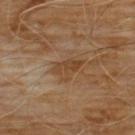Q: Is there a histopathology result?
A: catalogued during a skin exam; not biopsied
Q: How was this image acquired?
A: ~15 mm crop, total-body skin-cancer survey
Q: Where on the body is the lesion?
A: the chest
Q: Lesion size?
A: ~3 mm (longest diameter)
Q: Who is the patient?
A: male, aged 58 to 62
Q: Illumination type?
A: cross-polarized